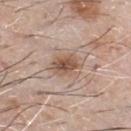Q: Is there a histopathology result?
A: imaged on a skin check; not biopsied
Q: Illumination type?
A: white-light
Q: How large is the lesion?
A: ≈3.5 mm
Q: What is the anatomic site?
A: the chest
Q: Automated lesion metrics?
A: about 12 CIELAB-L* units darker than the surrounding skin and a normalized lesion–skin contrast near 8.5
Q: What kind of image is this?
A: ~15 mm crop, total-body skin-cancer survey
Q: What are the patient's age and sex?
A: male, roughly 65 years of age